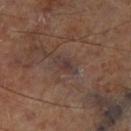| feature | finding |
|---|---|
| follow-up | imaged on a skin check; not biopsied |
| diameter | ~3 mm (longest diameter) |
| body site | the right lower leg |
| lighting | cross-polarized illumination |
| image | ~15 mm tile from a whole-body skin photo |